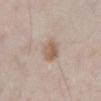Notes:
– workup · catalogued during a skin exam; not biopsied
– patient · male, aged 53–57
– imaging modality · ~15 mm tile from a whole-body skin photo
– lesion diameter · about 3 mm
– anatomic site · the abdomen
– tile lighting · white-light